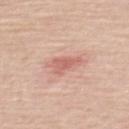From the upper back.
A male subject, roughly 60 years of age.
A roughly 15 mm field-of-view crop from a total-body skin photograph.
The recorded lesion diameter is about 3 mm.
Captured under white-light illumination.